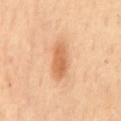automated metrics: internal color variation of about 3 on a 0–10 scale and radial color variation of about 1; subject: female, approximately 70 years of age; anatomic site: the mid back; lighting: cross-polarized; acquisition: total-body-photography crop, ~15 mm field of view; diameter: ~5 mm (longest diameter).